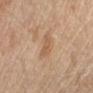Captured during whole-body skin photography for melanoma surveillance; the lesion was not biopsied. A female subject, approximately 45 years of age. Approximately 3 mm at its widest. On the right forearm. A roughly 15 mm field-of-view crop from a total-body skin photograph. Captured under cross-polarized illumination.